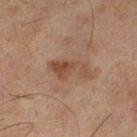{
  "site": "left lower leg",
  "patient": {
    "sex": "male",
    "age_approx": 45
  },
  "lesion_size": {
    "long_diameter_mm_approx": 4.5
  },
  "lighting": "cross-polarized",
  "image": {
    "source": "total-body photography crop",
    "field_of_view_mm": 15
  }
}